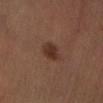Assessment:
No biopsy was performed on this lesion — it was imaged during a full skin examination and was not determined to be concerning.
Acquisition and patient details:
The lesion is on the left lower leg. Longest diameter approximately 2.5 mm. The patient is a male aged approximately 50. A roughly 15 mm field-of-view crop from a total-body skin photograph. The tile uses cross-polarized illumination. Automated image analysis of the tile measured an area of roughly 5 mm² and a shape-asymmetry score of about 0.15 (0 = symmetric). The software also gave a mean CIELAB color near L≈31 a*≈20 b*≈25, roughly 9 lightness units darker than nearby skin, and a normalized border contrast of about 8.5. It also reported a nevus-likeness score of about 95/100 and a detector confidence of about 100 out of 100 that the crop contains a lesion.